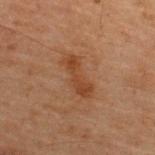biopsy_status: not biopsied; imaged during a skin examination
lesion_size:
  long_diameter_mm_approx: 5.0
image:
  source: total-body photography crop
  field_of_view_mm: 15
site: upper back
patient:
  sex: male
  age_approx: 60
automated_metrics:
  border_irregularity_0_10: 3.5
  peripheral_color_asymmetry: 0.5
lighting: cross-polarized